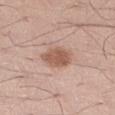follow-up — imaged on a skin check; not biopsied
automated metrics — a lesion area of about 9 mm², an outline eccentricity of about 0.55 (0 = round, 1 = elongated), and two-axis asymmetry of about 0.15; an average lesion color of about L≈57 a*≈21 b*≈28 (CIELAB), roughly 11 lightness units darker than nearby skin, and a lesion-to-skin contrast of about 8 (normalized; higher = more distinct); a border-irregularity rating of about 1.5/10, a color-variation rating of about 2.5/10, and peripheral color asymmetry of about 1; a nevus-likeness score of about 85/100
patient — male, aged 38–42
image source — 15 mm crop, total-body photography
location — the right thigh